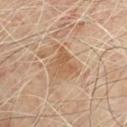Notes:
• acquisition — ~15 mm tile from a whole-body skin photo
• illumination — cross-polarized illumination
• diameter — about 3.5 mm
• location — the chest
• subject — male, aged approximately 70